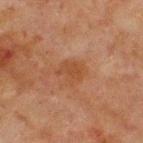Part of a total-body skin-imaging series; this lesion was reviewed on a skin check and was not flagged for biopsy. A 15 mm close-up extracted from a 3D total-body photography capture. The lesion is located on the front of the torso. The patient is a male about 65 years old. This is a cross-polarized tile. Longest diameter approximately 3 mm.